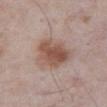This lesion was catalogued during total-body skin photography and was not selected for biopsy. A 15 mm close-up tile from a total-body photography series done for melanoma screening. A male subject in their mid-70s. This is a white-light tile. The lesion is on the abdomen.